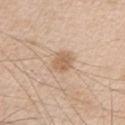Recorded during total-body skin imaging; not selected for excision or biopsy.
This is a white-light tile.
The lesion is located on the chest.
A male subject about 60 years old.
A region of skin cropped from a whole-body photographic capture, roughly 15 mm wide.